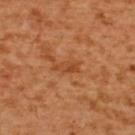Part of a total-body skin-imaging series; this lesion was reviewed on a skin check and was not flagged for biopsy. The subject is a female roughly 55 years of age. A lesion tile, about 15 mm wide, cut from a 3D total-body photograph. The lesion is on the upper back. This is a cross-polarized tile. The lesion-visualizer software estimated an area of roughly 4 mm², a shape eccentricity near 0.9, and a shape-asymmetry score of about 0.3 (0 = symmetric). And it measured a mean CIELAB color near L≈48 a*≈28 b*≈41, roughly 8 lightness units darker than nearby skin, and a normalized border contrast of about 6. It also reported an automated nevus-likeness rating near 0 out of 100 and lesion-presence confidence of about 100/100.